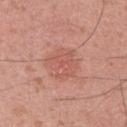Captured during whole-body skin photography for melanoma surveillance; the lesion was not biopsied.
Longest diameter approximately 4 mm.
The lesion is on the left upper arm.
A male subject aged approximately 35.
This image is a 15 mm lesion crop taken from a total-body photograph.
Captured under white-light illumination.